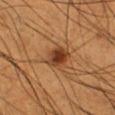Q: Is there a histopathology result?
A: catalogued during a skin exam; not biopsied
Q: What kind of image is this?
A: ~15 mm crop, total-body skin-cancer survey
Q: Patient demographics?
A: male, in their mid-50s
Q: What did automated image analysis measure?
A: an area of roughly 8.5 mm², an outline eccentricity of about 0.45 (0 = round, 1 = elongated), and a symmetry-axis asymmetry near 0.25; a mean CIELAB color near L≈41 a*≈23 b*≈35, roughly 12 lightness units darker than nearby skin, and a lesion-to-skin contrast of about 9.5 (normalized; higher = more distinct); a detector confidence of about 100 out of 100 that the crop contains a lesion
Q: What is the lesion's diameter?
A: ≈3.5 mm
Q: What lighting was used for the tile?
A: cross-polarized illumination
Q: Lesion location?
A: the leg